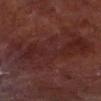Captured during whole-body skin photography for melanoma surveillance; the lesion was not biopsied.
This is a cross-polarized tile.
A region of skin cropped from a whole-body photographic capture, roughly 15 mm wide.
The total-body-photography lesion software estimated an area of roughly 44 mm² and a shape-asymmetry score of about 0.3 (0 = symmetric). And it measured a lesion-detection confidence of about 95/100.
A male patient aged around 70.
The lesion is located on the leg.
The recorded lesion diameter is about 11 mm.